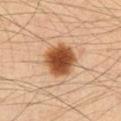Acquisition and patient details:
From the chest. Captured under cross-polarized illumination. A male subject aged around 35. This image is a 15 mm lesion crop taken from a total-body photograph. The lesion's longest dimension is about 4.5 mm. Automated tile analysis of the lesion measured an area of roughly 13 mm² and a symmetry-axis asymmetry near 0.15. The software also gave a lesion color around L≈48 a*≈23 b*≈36 in CIELAB, a lesion–skin lightness drop of about 18, and a normalized lesion–skin contrast near 13.